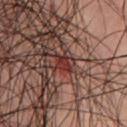workup=total-body-photography surveillance lesion; no biopsy
patient=male, in their mid- to late 40s
image=15 mm crop, total-body photography
site=the chest
tile lighting=cross-polarized